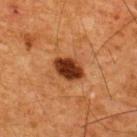biopsy status: total-body-photography surveillance lesion; no biopsy | image-analysis metrics: an outline eccentricity of about 0.75 (0 = round, 1 = elongated) and a symmetry-axis asymmetry near 0.2; a lesion color around L≈29 a*≈24 b*≈31 in CIELAB, a lesion–skin lightness drop of about 17, and a lesion-to-skin contrast of about 14.5 (normalized; higher = more distinct); a border-irregularity index near 2/10, internal color variation of about 3 on a 0–10 scale, and a peripheral color-asymmetry measure near 1 | size: about 3.5 mm | location: the upper back | image source: total-body-photography crop, ~15 mm field of view | lighting: cross-polarized | subject: male, aged 63 to 67.